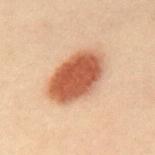Clinical impression: Captured during whole-body skin photography for melanoma surveillance; the lesion was not biopsied. Background: A 15 mm close-up extracted from a 3D total-body photography capture. The patient is a female aged 28 to 32. The lesion is located on the mid back. Automated image analysis of the tile measured a lesion area of about 23 mm², an eccentricity of roughly 0.8, and two-axis asymmetry of about 0.15. The software also gave a classifier nevus-likeness of about 100/100 and a lesion-detection confidence of about 100/100. About 7 mm across.